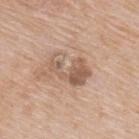Background: A region of skin cropped from a whole-body photographic capture, roughly 15 mm wide. The lesion is on the upper back. A male subject about 65 years old. The tile uses white-light illumination.